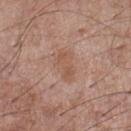The tile uses white-light illumination.
The lesion is on the left lower leg.
The lesion's longest dimension is about 4 mm.
A lesion tile, about 15 mm wide, cut from a 3D total-body photograph.
The total-body-photography lesion software estimated a lesion color around L≈54 a*≈20 b*≈28 in CIELAB and a lesion–skin lightness drop of about 6. The analysis additionally found border irregularity of about 3 on a 0–10 scale, a within-lesion color-variation index near 2/10, and a peripheral color-asymmetry measure near 0.5. And it measured a classifier nevus-likeness of about 0/100.
A male patient, in their mid- to late 50s.